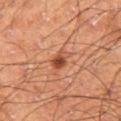Imaged during a routine full-body skin examination; the lesion was not biopsied and no histopathology is available.